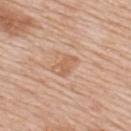The lesion was photographed on a routine skin check and not biopsied; there is no pathology result. A male patient, aged 58 to 62. From the back. Automated image analysis of the tile measured a footprint of about 4.5 mm², an eccentricity of roughly 0.8, and two-axis asymmetry of about 0.3. And it measured a detector confidence of about 100 out of 100 that the crop contains a lesion. This image is a 15 mm lesion crop taken from a total-body photograph. The tile uses white-light illumination. The recorded lesion diameter is about 3 mm.